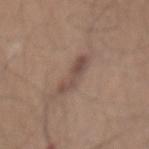Recorded during total-body skin imaging; not selected for excision or biopsy. The subject is a male roughly 40 years of age. On the mid back. A 15 mm close-up tile from a total-body photography series done for melanoma screening. Captured under white-light illumination. The recorded lesion diameter is about 4 mm.